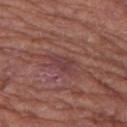{
  "biopsy_status": "not biopsied; imaged during a skin examination",
  "image": {
    "source": "total-body photography crop",
    "field_of_view_mm": 15
  },
  "automated_metrics": {
    "border_irregularity_0_10": 3.0,
    "color_variation_0_10": 1.5,
    "peripheral_color_asymmetry": 0.5,
    "nevus_likeness_0_100": 0
  },
  "site": "left thigh",
  "patient": {
    "sex": "male",
    "age_approx": 65
  },
  "lesion_size": {
    "long_diameter_mm_approx": 3.0
  },
  "lighting": "white-light"
}